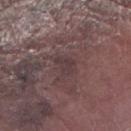No biopsy was performed on this lesion — it was imaged during a full skin examination and was not determined to be concerning.
Cropped from a total-body skin-imaging series; the visible field is about 15 mm.
The lesion is on the left forearm.
A male patient aged 73 to 77.